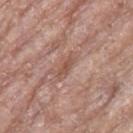Q: Was a biopsy performed?
A: imaged on a skin check; not biopsied
Q: What did automated image analysis measure?
A: roughly 9 lightness units darker than nearby skin; a detector confidence of about 55 out of 100 that the crop contains a lesion
Q: Where on the body is the lesion?
A: the right thigh
Q: Illumination type?
A: white-light illumination
Q: How was this image acquired?
A: 15 mm crop, total-body photography
Q: What are the patient's age and sex?
A: female, approximately 75 years of age
Q: What is the lesion's diameter?
A: ≈3 mm